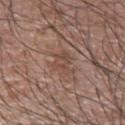The lesion was tiled from a total-body skin photograph and was not biopsied.